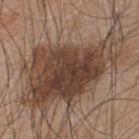biopsy status = no biopsy performed (imaged during a skin exam); location = the back; lesion diameter = ≈14.5 mm; image source = ~15 mm tile from a whole-body skin photo; lighting = white-light illumination; patient = male, aged around 45.